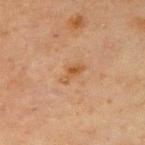Impression:
No biopsy was performed on this lesion — it was imaged during a full skin examination and was not determined to be concerning.
Background:
Cropped from a total-body skin-imaging series; the visible field is about 15 mm. Imaged with cross-polarized lighting. Measured at roughly 3 mm in maximum diameter. A male subject aged around 65. The lesion is on the left upper arm. Automated tile analysis of the lesion measured an eccentricity of roughly 0.85. And it measured a lesion color around L≈44 a*≈18 b*≈32 in CIELAB, a lesion–skin lightness drop of about 6, and a normalized lesion–skin contrast near 6.5.